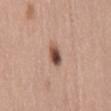This lesion was catalogued during total-body skin photography and was not selected for biopsy. Cropped from a whole-body photographic skin survey; the tile spans about 15 mm. A female subject roughly 40 years of age. The recorded lesion diameter is about 3 mm. The lesion is located on the mid back. Captured under white-light illumination.